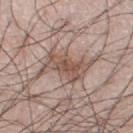Assessment: Recorded during total-body skin imaging; not selected for excision or biopsy. Image and clinical context: A 15 mm close-up extracted from a 3D total-body photography capture. A male patient, aged around 70. From the left leg.